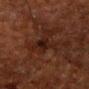Q: What is the anatomic site?
A: the head or neck
Q: What is the lesion's diameter?
A: ~4 mm (longest diameter)
Q: What is the imaging modality?
A: 15 mm crop, total-body photography
Q: Automated lesion metrics?
A: a lesion color around L≈14 a*≈16 b*≈17 in CIELAB and a lesion–skin lightness drop of about 5; a border-irregularity index near 4.5/10, internal color variation of about 4 on a 0–10 scale, and radial color variation of about 1.5
Q: Patient demographics?
A: male, approximately 60 years of age